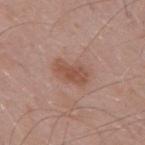Q: Patient demographics?
A: male, in their mid-50s
Q: What is the imaging modality?
A: total-body-photography crop, ~15 mm field of view
Q: Automated lesion metrics?
A: a mean CIELAB color near L≈52 a*≈21 b*≈28 and about 8 CIELAB-L* units darker than the surrounding skin; border irregularity of about 2.5 on a 0–10 scale, a color-variation rating of about 3/10, and peripheral color asymmetry of about 1; an automated nevus-likeness rating near 15 out of 100 and a detector confidence of about 100 out of 100 that the crop contains a lesion
Q: Where on the body is the lesion?
A: the mid back
Q: How was the tile lit?
A: white-light illumination